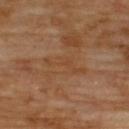workup — no biopsy performed (imaged during a skin exam)
acquisition — ~15 mm crop, total-body skin-cancer survey
patient — male, in their 70s
site — the back